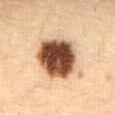Acquisition and patient details: Captured under cross-polarized illumination. A female subject, approximately 30 years of age. A 15 mm close-up extracted from a 3D total-body photography capture. The lesion is located on the abdomen.